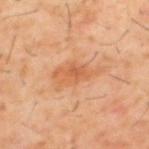Impression:
This lesion was catalogued during total-body skin photography and was not selected for biopsy.
Context:
The tile uses cross-polarized illumination. Cropped from a total-body skin-imaging series; the visible field is about 15 mm. A male patient, aged 58–62. The lesion is on the upper back. Automated tile analysis of the lesion measured a lesion–skin lightness drop of about 7. The software also gave lesion-presence confidence of about 100/100.